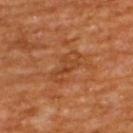The lesion was tiled from a total-body skin photograph and was not biopsied.
Automated tile analysis of the lesion measured two-axis asymmetry of about 0.35. It also reported a lesion–skin lightness drop of about 6. And it measured a border-irregularity index near 4.5/10 and a color-variation rating of about 2.5/10.
This image is a 15 mm lesion crop taken from a total-body photograph.
A male subject about 65 years old.
From the upper back.